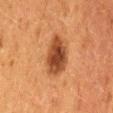notes: imaged on a skin check; not biopsied | location: the lower back | lesion size: ≈4.5 mm | image source: total-body-photography crop, ~15 mm field of view | automated lesion analysis: an area of roughly 13 mm² and an outline eccentricity of about 0.7 (0 = round, 1 = elongated) | patient: female, roughly 50 years of age | tile lighting: cross-polarized illumination.